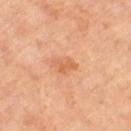{
  "biopsy_status": "not biopsied; imaged during a skin examination",
  "lighting": "cross-polarized",
  "image": {
    "source": "total-body photography crop",
    "field_of_view_mm": 15
  },
  "patient": {
    "sex": "female",
    "age_approx": 65
  },
  "site": "left thigh",
  "lesion_size": {
    "long_diameter_mm_approx": 2.5
  }
}